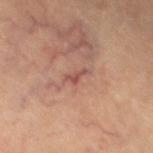Clinical impression:
Imaged during a routine full-body skin examination; the lesion was not biopsied and no histopathology is available.
Image and clinical context:
Longest diameter approximately 3 mm. A female patient, aged around 65. Automated tile analysis of the lesion measured an average lesion color of about L≈48 a*≈23 b*≈23 (CIELAB) and a lesion–skin lightness drop of about 8. And it measured a border-irregularity rating of about 4.5/10, internal color variation of about 0.5 on a 0–10 scale, and peripheral color asymmetry of about 0. The lesion is on the left thigh. Cropped from a whole-body photographic skin survey; the tile spans about 15 mm. Captured under cross-polarized illumination.